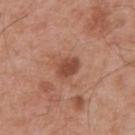Impression: No biopsy was performed on this lesion — it was imaged during a full skin examination and was not determined to be concerning. Acquisition and patient details: About 3 mm across. The patient is a male aged around 55. Captured under white-light illumination. Cropped from a total-body skin-imaging series; the visible field is about 15 mm. From the upper back.